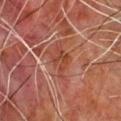Impression: Imaged during a routine full-body skin examination; the lesion was not biopsied and no histopathology is available. Acquisition and patient details: The lesion is located on the chest. A 15 mm close-up extracted from a 3D total-body photography capture. The patient is a male in their mid-60s.